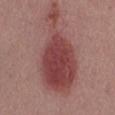Measured at roughly 12 mm in maximum diameter.
A male subject about 55 years old.
A lesion tile, about 15 mm wide, cut from a 3D total-body photograph.
Imaged with white-light lighting.
On the mid back.